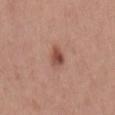follow-up — catalogued during a skin exam; not biopsied | size — ~2.5 mm (longest diameter) | tile lighting — white-light illumination | image — total-body-photography crop, ~15 mm field of view | body site — the mid back | patient — male, aged 28–32.